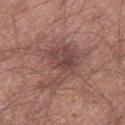Findings:
- automated metrics: an area of roughly 13 mm² and two-axis asymmetry of about 0.45; a mean CIELAB color near L≈45 a*≈20 b*≈22, roughly 8 lightness units darker than nearby skin, and a normalized lesion–skin contrast near 6.5; a border-irregularity index near 6/10, internal color variation of about 4.5 on a 0–10 scale, and peripheral color asymmetry of about 1.5; a classifier nevus-likeness of about 15/100 and a detector confidence of about 100 out of 100 that the crop contains a lesion
- illumination: white-light
- subject: male, aged 38–42
- image: ~15 mm crop, total-body skin-cancer survey
- anatomic site: the right thigh